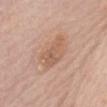Part of a total-body skin-imaging series; this lesion was reviewed on a skin check and was not flagged for biopsy.
Captured under white-light illumination.
A female subject in their mid-80s or older.
A 15 mm close-up extracted from a 3D total-body photography capture.
From the abdomen.
The lesion-visualizer software estimated an average lesion color of about L≈59 a*≈19 b*≈31 (CIELAB), a lesion–skin lightness drop of about 8, and a normalized border contrast of about 6. And it measured a border-irregularity index near 3/10 and a color-variation rating of about 3.5/10.
About 5 mm across.